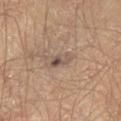This lesion was catalogued during total-body skin photography and was not selected for biopsy. The lesion is located on the left thigh. About 3 mm across. Imaged with cross-polarized lighting. A 15 mm close-up tile from a total-body photography series done for melanoma screening. The patient is a male aged around 65.